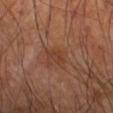The lesion was tiled from a total-body skin photograph and was not biopsied. A male patient, aged approximately 65. This image is a 15 mm lesion crop taken from a total-body photograph. Automated tile analysis of the lesion measured two-axis asymmetry of about 0.35. It also reported internal color variation of about 1.5 on a 0–10 scale and radial color variation of about 0.5. This is a cross-polarized tile. The lesion is located on the left forearm.